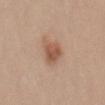workup: no biopsy performed (imaged during a skin exam); imaging modality: total-body-photography crop, ~15 mm field of view; automated metrics: a nevus-likeness score of about 95/100 and a lesion-detection confidence of about 100/100; diameter: ≈4 mm; site: the mid back; patient: female, in their mid-20s; tile lighting: white-light illumination.